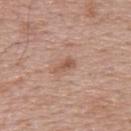Recorded during total-body skin imaging; not selected for excision or biopsy.
A 15 mm close-up tile from a total-body photography series done for melanoma screening.
A male subject, aged 68–72.
The total-body-photography lesion software estimated a lesion-detection confidence of about 100/100.
The tile uses white-light illumination.
The lesion is located on the upper back.